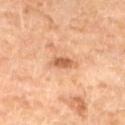Background: Approximately 3 mm at its widest. The subject is a female in their 70s. Located on the leg. A lesion tile, about 15 mm wide, cut from a 3D total-body photograph. This is a cross-polarized tile.